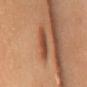Impression:
Captured during whole-body skin photography for melanoma surveillance; the lesion was not biopsied.
Background:
Captured under cross-polarized illumination. About 3.5 mm across. The lesion is located on the mid back. The subject is a female aged 48 to 52. A region of skin cropped from a whole-body photographic capture, roughly 15 mm wide.